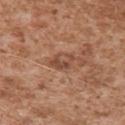No biopsy was performed on this lesion — it was imaged during a full skin examination and was not determined to be concerning.
Approximately 2.5 mm at its widest.
This is a white-light tile.
A close-up tile cropped from a whole-body skin photograph, about 15 mm across.
A male patient, in their mid- to late 40s.
Located on the right upper arm.
The total-body-photography lesion software estimated roughly 9 lightness units darker than nearby skin and a normalized border contrast of about 7. And it measured a border-irregularity rating of about 7.5/10. The analysis additionally found a nevus-likeness score of about 0/100 and a lesion-detection confidence of about 100/100.